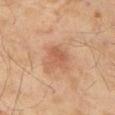biopsy status = imaged on a skin check; not biopsied
TBP lesion metrics = a mean CIELAB color near L≈52 a*≈22 b*≈31 and a normalized border contrast of about 5.5; a border-irregularity rating of about 2.5/10, a within-lesion color-variation index near 2.5/10, and radial color variation of about 0.5
tile lighting = cross-polarized
diameter = about 2.5 mm
acquisition = ~15 mm tile from a whole-body skin photo
location = the right thigh
patient = male, approximately 60 years of age